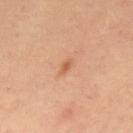Cropped from a whole-body photographic skin survey; the tile spans about 15 mm.
Approximately 2 mm at its widest.
A male patient aged approximately 60.
This is a cross-polarized tile.
The lesion is located on the upper back.
The total-body-photography lesion software estimated a mean CIELAB color near L≈57 a*≈24 b*≈36 and a normalized border contrast of about 6.5. The analysis additionally found a border-irregularity index near 2.5/10, a color-variation rating of about 0/10, and a peripheral color-asymmetry measure near 0. It also reported a nevus-likeness score of about 25/100 and lesion-presence confidence of about 100/100.